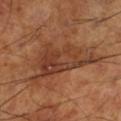Clinical impression:
Part of a total-body skin-imaging series; this lesion was reviewed on a skin check and was not flagged for biopsy.
Image and clinical context:
On the leg. This is a cross-polarized tile. Automated tile analysis of the lesion measured an average lesion color of about L≈40 a*≈23 b*≈31 (CIELAB), a lesion–skin lightness drop of about 9, and a normalized border contrast of about 7.5. The software also gave an automated nevus-likeness rating near 20 out of 100 and a detector confidence of about 95 out of 100 that the crop contains a lesion. A 15 mm close-up extracted from a 3D total-body photography capture. A male patient in their mid- to late 60s. Measured at roughly 10 mm in maximum diameter.